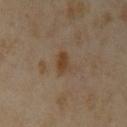Q: Lesion location?
A: the left upper arm
Q: What did automated image analysis measure?
A: a footprint of about 5 mm², an eccentricity of roughly 0.6, and a shape-asymmetry score of about 0.25 (0 = symmetric)
Q: Who is the patient?
A: female, aged around 35
Q: What is the imaging modality?
A: total-body-photography crop, ~15 mm field of view
Q: Illumination type?
A: cross-polarized